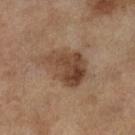Notes:
- workup: catalogued during a skin exam; not biopsied
- subject: female, aged 58 to 62
- diameter: ≈5.5 mm
- location: the left lower leg
- tile lighting: cross-polarized illumination
- acquisition: ~15 mm crop, total-body skin-cancer survey
- image-analysis metrics: border irregularity of about 3 on a 0–10 scale, a color-variation rating of about 6/10, and a peripheral color-asymmetry measure near 2.5; an automated nevus-likeness rating near 55 out of 100 and a detector confidence of about 100 out of 100 that the crop contains a lesion